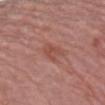Findings:
– biopsy status — no biopsy performed (imaged during a skin exam)
– subject — female, aged 63–67
– body site — the left forearm
– image source — 15 mm crop, total-body photography
– lighting — white-light illumination